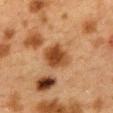Assessment: The lesion was photographed on a routine skin check and not biopsied; there is no pathology result. Background: A region of skin cropped from a whole-body photographic capture, roughly 15 mm wide. A female subject, aged 38–42. Imaged with cross-polarized lighting. Longest diameter approximately 4 mm. An algorithmic analysis of the crop reported a lesion color around L≈39 a*≈21 b*≈33 in CIELAB, roughly 12 lightness units darker than nearby skin, and a normalized lesion–skin contrast near 10. The lesion is located on the mid back.